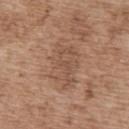Imaged during a routine full-body skin examination; the lesion was not biopsied and no histopathology is available. This is a white-light tile. Cropped from a whole-body photographic skin survey; the tile spans about 15 mm. The subject is a male roughly 70 years of age. Located on the upper back. Longest diameter approximately 6.5 mm. Automated image analysis of the tile measured a mean CIELAB color near L≈52 a*≈18 b*≈29, roughly 7 lightness units darker than nearby skin, and a lesion-to-skin contrast of about 5 (normalized; higher = more distinct). It also reported a classifier nevus-likeness of about 0/100.